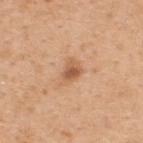{"biopsy_status": "not biopsied; imaged during a skin examination", "lighting": "white-light", "site": "upper back", "lesion_size": {"long_diameter_mm_approx": 2.5}, "patient": {"sex": "male", "age_approx": 50}, "image": {"source": "total-body photography crop", "field_of_view_mm": 15}}